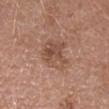notes = no biopsy performed (imaged during a skin exam) | acquisition = 15 mm crop, total-body photography | site = the arm | subject = female, in their mid- to late 40s | TBP lesion metrics = a mean CIELAB color near L≈50 a*≈20 b*≈28 and a normalized border contrast of about 6; a within-lesion color-variation index near 5.5/10 and peripheral color asymmetry of about 2.5 | illumination = white-light | diameter = ~4 mm (longest diameter).